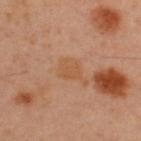follow-up=total-body-photography surveillance lesion; no biopsy | body site=the upper back | lesion diameter=about 2.5 mm | automated metrics=a lesion color around L≈52 a*≈21 b*≈35 in CIELAB and about 5 CIELAB-L* units darker than the surrounding skin | image source=~15 mm tile from a whole-body skin photo | patient=male, roughly 45 years of age | lighting=cross-polarized.